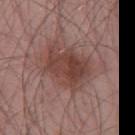biopsy status: imaged on a skin check; not biopsied | location: the left thigh | tile lighting: white-light | size: ~6 mm (longest diameter) | imaging modality: ~15 mm crop, total-body skin-cancer survey | patient: male, aged 53–57 | automated metrics: a footprint of about 21 mm²; a lesion–skin lightness drop of about 9 and a normalized lesion–skin contrast near 8; a border-irregularity index near 3.5/10, internal color variation of about 5 on a 0–10 scale, and peripheral color asymmetry of about 1.5.